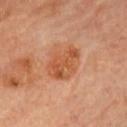* notes · imaged on a skin check; not biopsied
* image source · ~15 mm tile from a whole-body skin photo
* site · the chest
* patient · male, aged approximately 65